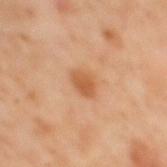Findings:
* notes: catalogued during a skin exam; not biopsied
* patient: male, approximately 60 years of age
* site: the mid back
* image: 15 mm crop, total-body photography
* TBP lesion metrics: about 9 CIELAB-L* units darker than the surrounding skin; a border-irregularity rating of about 1.5/10, a color-variation rating of about 2/10, and a peripheral color-asymmetry measure near 0.5